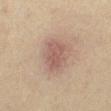| feature | finding |
|---|---|
| follow-up | catalogued during a skin exam; not biopsied |
| acquisition | 15 mm crop, total-body photography |
| subject | female, aged approximately 40 |
| image-analysis metrics | a mean CIELAB color near L≈46 a*≈16 b*≈21, a lesion–skin lightness drop of about 8, and a lesion-to-skin contrast of about 6.5 (normalized; higher = more distinct); a border-irregularity rating of about 2/10, a within-lesion color-variation index near 2/10, and a peripheral color-asymmetry measure near 0.5 |
| lesion diameter | about 3.5 mm |
| illumination | cross-polarized illumination |
| body site | the left lower leg |